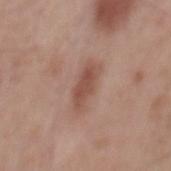<record>
  <biopsy_status>not biopsied; imaged during a skin examination</biopsy_status>
  <automated_metrics>
    <border_irregularity_0_10>3.5</border_irregularity_0_10>
    <peripheral_color_asymmetry>0.5</peripheral_color_asymmetry>
    <nevus_likeness_0_100>20</nevus_likeness_0_100>
    <lesion_detection_confidence_0_100>100</lesion_detection_confidence_0_100>
  </automated_metrics>
  <patient>
    <sex>male</sex>
    <age_approx>55</age_approx>
  </patient>
  <site>mid back</site>
  <lighting>white-light</lighting>
  <image>
    <source>total-body photography crop</source>
    <field_of_view_mm>15</field_of_view_mm>
  </image>
  <lesion_size>
    <long_diameter_mm_approx>4.5</long_diameter_mm_approx>
  </lesion_size>
</record>